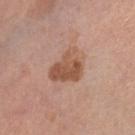biopsy status: catalogued during a skin exam; not biopsied